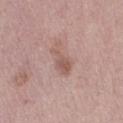- notes: no biopsy performed (imaged during a skin exam)
- illumination: white-light
- subject: female, about 50 years old
- anatomic site: the leg
- image source: ~15 mm crop, total-body skin-cancer survey
- diameter: ≈4.5 mm
- automated metrics: about 9 CIELAB-L* units darker than the surrounding skin and a lesion-to-skin contrast of about 6 (normalized; higher = more distinct); a classifier nevus-likeness of about 0/100 and a detector confidence of about 100 out of 100 that the crop contains a lesion